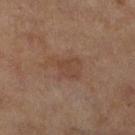follow-up: no biopsy performed (imaged during a skin exam) | patient: female, aged around 60 | acquisition: ~15 mm tile from a whole-body skin photo | body site: the leg | automated metrics: an area of roughly 8 mm² and an outline eccentricity of about 0.75 (0 = round, 1 = elongated); an average lesion color of about L≈37 a*≈16 b*≈24 (CIELAB), about 5 CIELAB-L* units darker than the surrounding skin, and a normalized lesion–skin contrast near 5; an automated nevus-likeness rating near 0 out of 100 and a lesion-detection confidence of about 100/100.